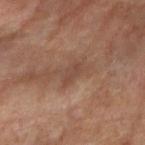Assessment:
This lesion was catalogued during total-body skin photography and was not selected for biopsy.
Clinical summary:
Approximately 3 mm at its widest. The tile uses cross-polarized illumination. A roughly 15 mm field-of-view crop from a total-body skin photograph. A female patient, approximately 50 years of age. Automated tile analysis of the lesion measured a lesion area of about 3 mm², a shape eccentricity near 0.85, and a symmetry-axis asymmetry near 0.35. The analysis additionally found a mean CIELAB color near L≈43 a*≈19 b*≈26, about 6 CIELAB-L* units darker than the surrounding skin, and a normalized lesion–skin contrast near 5.5. The software also gave a border-irregularity rating of about 3/10 and a within-lesion color-variation index near 1/10. The lesion is located on the right forearm.